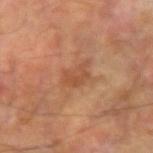The lesion was photographed on a routine skin check and not biopsied; there is no pathology result.
A male patient, aged approximately 70.
The tile uses cross-polarized illumination.
The lesion's longest dimension is about 3.5 mm.
Located on the left forearm.
A close-up tile cropped from a whole-body skin photograph, about 15 mm across.